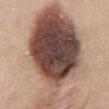– notes: catalogued during a skin exam; not biopsied
– location: the abdomen
– patient: female, aged approximately 40
– image: ~15 mm crop, total-body skin-cancer survey
– lesion size: about 16 mm